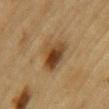Captured during whole-body skin photography for melanoma surveillance; the lesion was not biopsied. Longest diameter approximately 4 mm. From the arm. Cropped from a total-body skin-imaging series; the visible field is about 15 mm. A male patient, in their mid-80s or older.